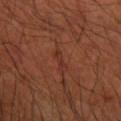No biopsy was performed on this lesion — it was imaged during a full skin examination and was not determined to be concerning. A male subject aged around 65. Measured at roughly 2.5 mm in maximum diameter. A lesion tile, about 15 mm wide, cut from a 3D total-body photograph. Automated image analysis of the tile measured radial color variation of about 0. On the arm.